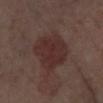Clinical impression:
Recorded during total-body skin imaging; not selected for excision or biopsy.
Clinical summary:
A 15 mm crop from a total-body photograph taken for skin-cancer surveillance. The lesion is on the right lower leg. A female patient aged 53 to 57. Approximately 6.5 mm at its widest. Automated image analysis of the tile measured a shape eccentricity near 0.8 and a symmetry-axis asymmetry near 0.2. And it measured a mean CIELAB color near L≈29 a*≈18 b*≈19, a lesion–skin lightness drop of about 7, and a lesion-to-skin contrast of about 7.5 (normalized; higher = more distinct). The analysis additionally found internal color variation of about 2.5 on a 0–10 scale and peripheral color asymmetry of about 1. And it measured a classifier nevus-likeness of about 45/100. Captured under cross-polarized illumination.